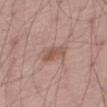follow-up: total-body-photography surveillance lesion; no biopsy | automated lesion analysis: a footprint of about 5.5 mm²; an average lesion color of about L≈54 a*≈20 b*≈26 (CIELAB) and a normalized lesion–skin contrast near 6.5; border irregularity of about 3 on a 0–10 scale and radial color variation of about 1; a classifier nevus-likeness of about 30/100 and a detector confidence of about 100 out of 100 that the crop contains a lesion | subject: male, in their 60s | location: the right thigh | acquisition: total-body-photography crop, ~15 mm field of view.